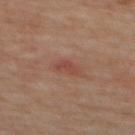Recorded during total-body skin imaging; not selected for excision or biopsy. The patient is a male approximately 60 years of age. The lesion is located on the back. Cropped from a whole-body photographic skin survey; the tile spans about 15 mm. The recorded lesion diameter is about 2.5 mm.